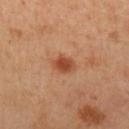notes: catalogued during a skin exam; not biopsied
automated metrics: an automated nevus-likeness rating near 85 out of 100 and a lesion-detection confidence of about 100/100
tile lighting: cross-polarized
image: 15 mm crop, total-body photography
diameter: ≈3 mm
subject: female, approximately 40 years of age
body site: the left upper arm